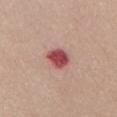Imaged during a routine full-body skin examination; the lesion was not biopsied and no histopathology is available. Cropped from a whole-body photographic skin survey; the tile spans about 15 mm. The lesion is on the front of the torso. The patient is a female about 45 years old.